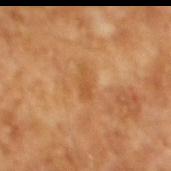Captured during whole-body skin photography for melanoma surveillance; the lesion was not biopsied.
The lesion-visualizer software estimated an area of roughly 2.5 mm² and an eccentricity of roughly 0.9. And it measured a lesion-detection confidence of about 100/100.
Captured under cross-polarized illumination.
A male patient, aged 63 to 67.
Measured at roughly 2.5 mm in maximum diameter.
A 15 mm close-up tile from a total-body photography series done for melanoma screening.